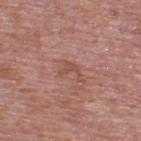No biopsy was performed on this lesion — it was imaged during a full skin examination and was not determined to be concerning. The tile uses white-light illumination. The total-body-photography lesion software estimated roughly 7 lightness units darker than nearby skin. The analysis additionally found a border-irregularity rating of about 7.5/10. A male patient aged 73–77. A region of skin cropped from a whole-body photographic capture, roughly 15 mm wide. Located on the upper back. The recorded lesion diameter is about 2.5 mm.